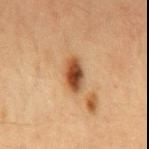The lesion is on the mid back.
Cropped from a total-body skin-imaging series; the visible field is about 15 mm.
The lesion's longest dimension is about 4 mm.
A male patient, aged around 65.
Imaged with cross-polarized lighting.